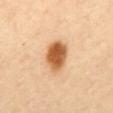- biopsy status — total-body-photography surveillance lesion; no biopsy
- illumination — cross-polarized illumination
- location — the back
- lesion size — ≈4 mm
- imaging modality — total-body-photography crop, ~15 mm field of view
- patient — female, aged 58–62